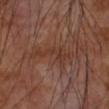Assessment: This lesion was catalogued during total-body skin photography and was not selected for biopsy. Image and clinical context: Cropped from a total-body skin-imaging series; the visible field is about 15 mm. The lesion is located on the left forearm. A male patient, aged 68 to 72. Captured under cross-polarized illumination.